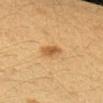biopsy status: no biopsy performed (imaged during a skin exam).